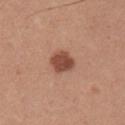workup: no biopsy performed (imaged during a skin exam); lesion diameter: about 3 mm; patient: male, about 35 years old; lighting: white-light; image: ~15 mm crop, total-body skin-cancer survey; body site: the arm.